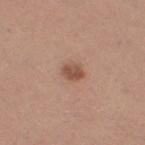Q: Was this lesion biopsied?
A: imaged on a skin check; not biopsied
Q: Automated lesion metrics?
A: an area of roughly 4 mm², an outline eccentricity of about 0.6 (0 = round, 1 = elongated), and a shape-asymmetry score of about 0.25 (0 = symmetric); a lesion color around L≈51 a*≈22 b*≈29 in CIELAB; border irregularity of about 2.5 on a 0–10 scale, a within-lesion color-variation index near 2/10, and peripheral color asymmetry of about 1
Q: Lesion location?
A: the right thigh
Q: How was this image acquired?
A: total-body-photography crop, ~15 mm field of view
Q: How large is the lesion?
A: about 2.5 mm
Q: What are the patient's age and sex?
A: female, aged around 30